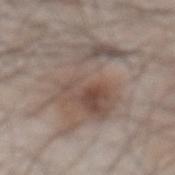Recorded during total-body skin imaging; not selected for excision or biopsy.
An algorithmic analysis of the crop reported an area of roughly 30 mm² and two-axis asymmetry of about 0.6. And it measured a border-irregularity index near 9.5/10, a color-variation rating of about 8/10, and radial color variation of about 3.
On the front of the torso.
The tile uses white-light illumination.
A 15 mm close-up tile from a total-body photography series done for melanoma screening.
Longest diameter approximately 9 mm.
The patient is a male aged 53 to 57.